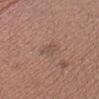Imaged during a routine full-body skin examination; the lesion was not biopsied and no histopathology is available. A male patient roughly 35 years of age. Located on the head or neck. Cropped from a whole-body photographic skin survey; the tile spans about 15 mm. The tile uses white-light illumination. Measured at roughly 3 mm in maximum diameter.